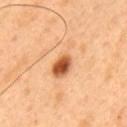| field | value |
|---|---|
| biopsy status | imaged on a skin check; not biopsied |
| site | the mid back |
| subject | male, in their 50s |
| lesion size | ≈6 mm |
| image source | 15 mm crop, total-body photography |
| TBP lesion metrics | an average lesion color of about L≈64 a*≈24 b*≈40 (CIELAB), roughly 12 lightness units darker than nearby skin, and a normalized border contrast of about 7.5; a border-irregularity rating of about 5/10, a color-variation rating of about 10/10, and a peripheral color-asymmetry measure near 3.5 |